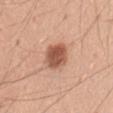Impression:
Imaged during a routine full-body skin examination; the lesion was not biopsied and no histopathology is available.
Context:
On the abdomen. About 3.5 mm across. Cropped from a total-body skin-imaging series; the visible field is about 15 mm. The subject is a male roughly 50 years of age. The tile uses white-light illumination.